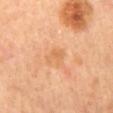This lesion was catalogued during total-body skin photography and was not selected for biopsy. From the back. Captured under cross-polarized illumination. A female subject aged around 65. Cropped from a whole-body photographic skin survey; the tile spans about 15 mm. About 2.5 mm across.